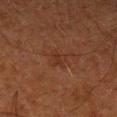biopsy status = imaged on a skin check; not biopsied | image = ~15 mm crop, total-body skin-cancer survey | patient = male, aged around 80 | anatomic site = the left lower leg.